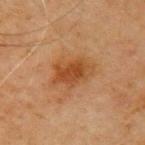  biopsy_status: not biopsied; imaged during a skin examination
  lesion_size:
    long_diameter_mm_approx: 4.5
  site: right upper arm
  automated_metrics:
    nevus_likeness_0_100: 80
    lesion_detection_confidence_0_100: 100
  image:
    source: total-body photography crop
    field_of_view_mm: 15
  patient:
    sex: male
    age_approx: 70